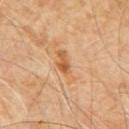Notes:
- notes — imaged on a skin check; not biopsied
- illumination — cross-polarized
- location — the front of the torso
- subject — male, aged 58 to 62
- image source — total-body-photography crop, ~15 mm field of view